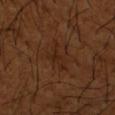The lesion was tiled from a total-body skin photograph and was not biopsied. This image is a 15 mm lesion crop taken from a total-body photograph. On the left forearm. The subject is a male aged approximately 65.